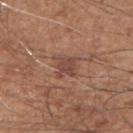<record>
<biopsy_status>not biopsied; imaged during a skin examination</biopsy_status>
<lesion_size>
  <long_diameter_mm_approx>2.5</long_diameter_mm_approx>
</lesion_size>
<site>right upper arm</site>
<automated_metrics>
  <cielab_L>45</cielab_L>
  <cielab_a>22</cielab_a>
  <cielab_b>26</cielab_b>
  <vs_skin_darker_L>8.0</vs_skin_darker_L>
</automated_metrics>
<image>
  <source>total-body photography crop</source>
  <field_of_view_mm>15</field_of_view_mm>
</image>
<patient>
  <sex>male</sex>
  <age_approx>65</age_approx>
</patient>
</record>